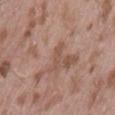{"biopsy_status": "not biopsied; imaged during a skin examination", "automated_metrics": {"cielab_L": 52, "cielab_a": 19, "cielab_b": 27, "vs_skin_darker_L": 7.0, "border_irregularity_0_10": 5.0, "color_variation_0_10": 0.0, "peripheral_color_asymmetry": 0.0}, "lesion_size": {"long_diameter_mm_approx": 3.0}, "image": {"source": "total-body photography crop", "field_of_view_mm": 15}, "site": "lower back", "patient": {"sex": "male", "age_approx": 55}, "lighting": "white-light"}